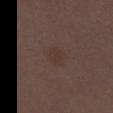The tile uses white-light illumination.
The lesion is on the mid back.
A roughly 15 mm field-of-view crop from a total-body skin photograph.
Longest diameter approximately 3 mm.
The patient is a female aged 28–32.